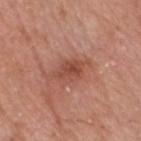Clinical impression:
Part of a total-body skin-imaging series; this lesion was reviewed on a skin check and was not flagged for biopsy.
Background:
The lesion-visualizer software estimated a mean CIELAB color near L≈48 a*≈26 b*≈29. A close-up tile cropped from a whole-body skin photograph, about 15 mm across. The patient is a male approximately 80 years of age. On the head or neck. Imaged with white-light lighting. Longest diameter approximately 3.5 mm.